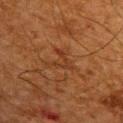Recorded during total-body skin imaging; not selected for excision or biopsy. From the upper back. A lesion tile, about 15 mm wide, cut from a 3D total-body photograph. A male subject, about 65 years old.